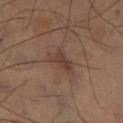{
  "biopsy_status": "not biopsied; imaged during a skin examination",
  "automated_metrics": {
    "area_mm2_approx": 6.0,
    "eccentricity": 0.85,
    "shape_asymmetry": 0.45,
    "border_irregularity_0_10": 4.5,
    "peripheral_color_asymmetry": 0.5
  },
  "site": "left lower leg",
  "image": {
    "source": "total-body photography crop",
    "field_of_view_mm": 15
  },
  "lighting": "cross-polarized",
  "patient": {
    "sex": "male",
    "age_approx": 60
  },
  "lesion_size": {
    "long_diameter_mm_approx": 4.0
  }
}